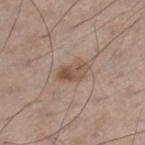Q: Patient demographics?
A: male, aged 58–62
Q: What is the lesion's diameter?
A: ~4 mm (longest diameter)
Q: What is the imaging modality?
A: ~15 mm crop, total-body skin-cancer survey
Q: Illumination type?
A: white-light illumination
Q: What is the anatomic site?
A: the right lower leg